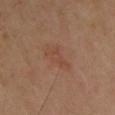Imaged during a routine full-body skin examination; the lesion was not biopsied and no histopathology is available.
A male subject approximately 55 years of age.
A roughly 15 mm field-of-view crop from a total-body skin photograph.
Located on the chest.